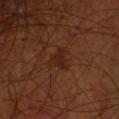Background:
An algorithmic analysis of the crop reported an area of roughly 5 mm², a shape eccentricity near 0.65, and two-axis asymmetry of about 0.55. And it measured an average lesion color of about L≈22 a*≈19 b*≈26 (CIELAB), roughly 5 lightness units darker than nearby skin, and a lesion-to-skin contrast of about 6 (normalized; higher = more distinct). And it measured a border-irregularity index near 5/10, internal color variation of about 1.5 on a 0–10 scale, and a peripheral color-asymmetry measure near 0.5. It also reported a nevus-likeness score of about 5/100 and a detector confidence of about 100 out of 100 that the crop contains a lesion. The lesion's longest dimension is about 3 mm. The lesion is located on the left forearm. The subject is a male in their 70s. A 15 mm close-up extracted from a 3D total-body photography capture. Captured under cross-polarized illumination.